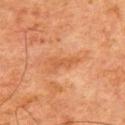Case summary:
- subject · male, aged around 80
- location · the chest
- imaging modality · ~15 mm crop, total-body skin-cancer survey